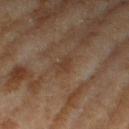Q: What are the patient's age and sex?
A: female, roughly 60 years of age
Q: What kind of image is this?
A: 15 mm crop, total-body photography
Q: Illumination type?
A: cross-polarized illumination
Q: Lesion location?
A: the right thigh
Q: Automated lesion metrics?
A: a footprint of about 2.5 mm², an eccentricity of roughly 0.85, and a shape-asymmetry score of about 0.3 (0 = symmetric); a lesion color around L≈34 a*≈16 b*≈26 in CIELAB; an automated nevus-likeness rating near 0 out of 100